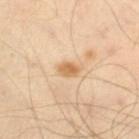Q: Is there a histopathology result?
A: no biopsy performed (imaged during a skin exam)
Q: Illumination type?
A: cross-polarized illumination
Q: Where on the body is the lesion?
A: the right lower leg
Q: What is the imaging modality?
A: ~15 mm crop, total-body skin-cancer survey
Q: What did automated image analysis measure?
A: a lesion area of about 3.5 mm², a shape eccentricity near 0.7, and two-axis asymmetry of about 0.2; a mean CIELAB color near L≈66 a*≈19 b*≈40, a lesion–skin lightness drop of about 11, and a normalized lesion–skin contrast near 8
Q: What are the patient's age and sex?
A: male, approximately 55 years of age